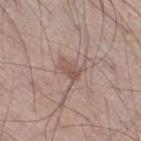Imaged during a routine full-body skin examination; the lesion was not biopsied and no histopathology is available. A male patient aged approximately 55. The recorded lesion diameter is about 2.5 mm. Automated tile analysis of the lesion measured a color-variation rating of about 2/10. The tile uses white-light illumination. A 15 mm close-up extracted from a 3D total-body photography capture. From the right thigh.